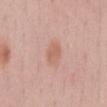The lesion was photographed on a routine skin check and not biopsied; there is no pathology result. A male patient aged 58 to 62. The total-body-photography lesion software estimated an area of roughly 4.5 mm², an eccentricity of roughly 0.8, and a symmetry-axis asymmetry near 0.2. It also reported an average lesion color of about L≈61 a*≈21 b*≈30 (CIELAB) and a lesion–skin lightness drop of about 7. And it measured a classifier nevus-likeness of about 25/100 and lesion-presence confidence of about 100/100. Located on the chest. A roughly 15 mm field-of-view crop from a total-body skin photograph. The tile uses white-light illumination. Longest diameter approximately 3 mm.